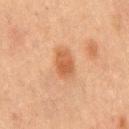Case summary:
– workup — no biopsy performed (imaged during a skin exam)
– anatomic site — the mid back
– TBP lesion metrics — an area of roughly 7 mm², an eccentricity of roughly 0.8, and a symmetry-axis asymmetry near 0.2
– subject — male, in their mid-60s
– size — about 4 mm
– acquisition — 15 mm crop, total-body photography